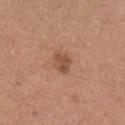{
  "biopsy_status": "not biopsied; imaged during a skin examination",
  "automated_metrics": {
    "eccentricity": 0.65,
    "shape_asymmetry": 0.2,
    "color_variation_0_10": 2.0,
    "peripheral_color_asymmetry": 1.0,
    "nevus_likeness_0_100": 40,
    "lesion_detection_confidence_0_100": 100
  },
  "lesion_size": {
    "long_diameter_mm_approx": 3.0
  },
  "patient": {
    "sex": "male",
    "age_approx": 35
  },
  "lighting": "white-light",
  "image": {
    "source": "total-body photography crop",
    "field_of_view_mm": 15
  },
  "site": "right upper arm"
}